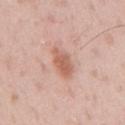Q: Was this lesion biopsied?
A: imaged on a skin check; not biopsied
Q: What is the imaging modality?
A: ~15 mm crop, total-body skin-cancer survey
Q: Lesion size?
A: ~4 mm (longest diameter)
Q: Illumination type?
A: white-light
Q: Where on the body is the lesion?
A: the right upper arm
Q: Patient demographics?
A: male, approximately 40 years of age